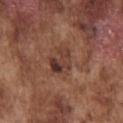Captured during whole-body skin photography for melanoma surveillance; the lesion was not biopsied. A region of skin cropped from a whole-body photographic capture, roughly 15 mm wide. The lesion's longest dimension is about 3.5 mm. Located on the chest. Imaged with white-light lighting. A male subject, aged 73 to 77. Automated image analysis of the tile measured a border-irregularity index near 4/10, internal color variation of about 9 on a 0–10 scale, and peripheral color asymmetry of about 3. And it measured a classifier nevus-likeness of about 0/100 and a lesion-detection confidence of about 100/100.